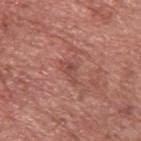The subject is a male aged 73 to 77.
Located on the upper back.
Cropped from a total-body skin-imaging series; the visible field is about 15 mm.
Imaged with white-light lighting.
The lesion's longest dimension is about 3 mm.